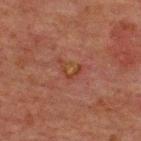biopsy_status: not biopsied; imaged during a skin examination